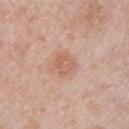Notes:
- notes — imaged on a skin check; not biopsied
- TBP lesion metrics — a lesion area of about 4.5 mm², a shape eccentricity near 0.6, and a shape-asymmetry score of about 0.2 (0 = symmetric); a lesion color around L≈60 a*≈22 b*≈30 in CIELAB, roughly 8 lightness units darker than nearby skin, and a normalized lesion–skin contrast near 6; internal color variation of about 2.5 on a 0–10 scale and peripheral color asymmetry of about 1; a classifier nevus-likeness of about 70/100 and a lesion-detection confidence of about 100/100
- anatomic site — the left thigh
- acquisition — total-body-photography crop, ~15 mm field of view
- subject — female, approximately 60 years of age